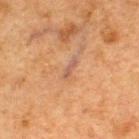Impression:
Recorded during total-body skin imaging; not selected for excision or biopsy.
Context:
The recorded lesion diameter is about 2.5 mm. The lesion is on the upper back. A male subject aged 48 to 52. Automated tile analysis of the lesion measured a footprint of about 2 mm², an outline eccentricity of about 0.95 (0 = round, 1 = elongated), and a symmetry-axis asymmetry near 0.3. It also reported a lesion color around L≈44 a*≈18 b*≈25 in CIELAB, roughly 7 lightness units darker than nearby skin, and a lesion-to-skin contrast of about 7 (normalized; higher = more distinct). The analysis additionally found a classifier nevus-likeness of about 0/100. This is a cross-polarized tile. A region of skin cropped from a whole-body photographic capture, roughly 15 mm wide.